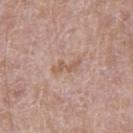Clinical impression:
The lesion was photographed on a routine skin check and not biopsied; there is no pathology result.
Acquisition and patient details:
A male subject, aged around 65. A 15 mm close-up extracted from a 3D total-body photography capture. The lesion-visualizer software estimated a border-irregularity index near 8/10, a within-lesion color-variation index near 0/10, and peripheral color asymmetry of about 0. The analysis additionally found a nevus-likeness score of about 0/100. The lesion is located on the left thigh. Measured at roughly 3.5 mm in maximum diameter.